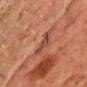Captured during whole-body skin photography for melanoma surveillance; the lesion was not biopsied.
A 15 mm crop from a total-body photograph taken for skin-cancer surveillance.
A male patient aged approximately 60.
The lesion is on the chest.